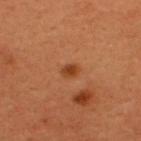Q: What did automated image analysis measure?
A: a lesion color around L≈40 a*≈27 b*≈38 in CIELAB, a lesion–skin lightness drop of about 10, and a lesion-to-skin contrast of about 8.5 (normalized; higher = more distinct); a color-variation rating of about 2/10 and radial color variation of about 0.5; a nevus-likeness score of about 85/100
Q: How large is the lesion?
A: ~2 mm (longest diameter)
Q: What is the imaging modality?
A: total-body-photography crop, ~15 mm field of view
Q: Who is the patient?
A: male, roughly 65 years of age
Q: What is the anatomic site?
A: the upper back